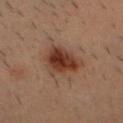<tbp_lesion>
<biopsy_status>not biopsied; imaged during a skin examination</biopsy_status>
<patient>
  <sex>male</sex>
  <age_approx>30</age_approx>
</patient>
<lesion_size>
  <long_diameter_mm_approx>4.5</long_diameter_mm_approx>
</lesion_size>
<automated_metrics>
  <vs_skin_darker_L>10.0</vs_skin_darker_L>
  <vs_skin_contrast_norm>10.5</vs_skin_contrast_norm>
  <border_irregularity_0_10>3.0</border_irregularity_0_10>
  <color_variation_0_10>5.0</color_variation_0_10>
  <peripheral_color_asymmetry>1.5</peripheral_color_asymmetry>
</automated_metrics>
<image>
  <source>total-body photography crop</source>
  <field_of_view_mm>15</field_of_view_mm>
</image>
<lighting>cross-polarized</lighting>
<site>head or neck</site>
</tbp_lesion>